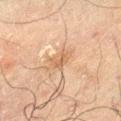notes: total-body-photography surveillance lesion; no biopsy
imaging modality: ~15 mm tile from a whole-body skin photo
tile lighting: cross-polarized illumination
site: the leg
subject: male, in their 70s
diameter: about 2.5 mm